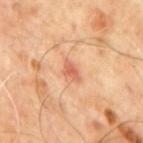Case summary:
– biopsy status: catalogued during a skin exam; not biopsied
– patient: male, aged 63 to 67
– body site: the mid back
– acquisition: total-body-photography crop, ~15 mm field of view
– lesion diameter: ~3 mm (longest diameter)